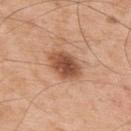notes: no biopsy performed (imaged during a skin exam) | patient: male, about 55 years old | anatomic site: the upper back | lighting: white-light illumination | imaging modality: ~15 mm crop, total-body skin-cancer survey | automated metrics: two-axis asymmetry of about 0.15; a mean CIELAB color near L≈51 a*≈24 b*≈33, about 15 CIELAB-L* units darker than the surrounding skin, and a lesion-to-skin contrast of about 10 (normalized; higher = more distinct) | lesion diameter: ≈4 mm.